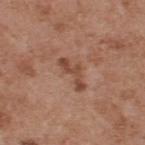No biopsy was performed on this lesion — it was imaged during a full skin examination and was not determined to be concerning. A 15 mm close-up extracted from a 3D total-body photography capture. The lesion is located on the back. The recorded lesion diameter is about 4 mm. A male subject, approximately 55 years of age. The lesion-visualizer software estimated a footprint of about 5 mm², a shape eccentricity near 0.9, and a symmetry-axis asymmetry near 0.6. And it measured a mean CIELAB color near L≈47 a*≈22 b*≈29, about 10 CIELAB-L* units darker than the surrounding skin, and a lesion-to-skin contrast of about 7.5 (normalized; higher = more distinct). Captured under white-light illumination.